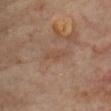Assessment:
Part of a total-body skin-imaging series; this lesion was reviewed on a skin check and was not flagged for biopsy.
Context:
A roughly 15 mm field-of-view crop from a total-body skin photograph. The subject is a female aged 58–62. The lesion's longest dimension is about 3.5 mm. Located on the chest.